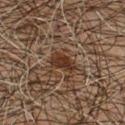Clinical impression: Recorded during total-body skin imaging; not selected for excision or biopsy.